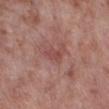{"biopsy_status": "not biopsied; imaged during a skin examination", "patient": {"sex": "male", "age_approx": 55}, "lesion_size": {"long_diameter_mm_approx": 3.5}, "site": "right lower leg", "lighting": "white-light", "image": {"source": "total-body photography crop", "field_of_view_mm": 15}}